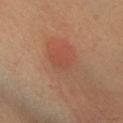The lesion was photographed on a routine skin check and not biopsied; there is no pathology result. A female subject, about 50 years old. This is a cross-polarized tile. The recorded lesion diameter is about 2.5 mm. A lesion tile, about 15 mm wide, cut from a 3D total-body photograph. The lesion is located on the right lower leg.